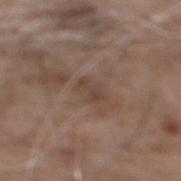Image and clinical context:
A 15 mm close-up tile from a total-body photography series done for melanoma screening. Automated image analysis of the tile measured a lesion area of about 3.5 mm², an eccentricity of roughly 0.75, and two-axis asymmetry of about 0.35. And it measured a mean CIELAB color near L≈42 a*≈16 b*≈24. The analysis additionally found peripheral color asymmetry of about 0. Imaged with white-light lighting. A male subject, roughly 60 years of age. The lesion is on the back.